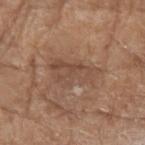The subject is a female approximately 75 years of age.
This is a white-light tile.
A 15 mm close-up tile from a total-body photography series done for melanoma screening.
The lesion is located on the right forearm.
The recorded lesion diameter is about 6 mm.